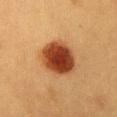The tile uses cross-polarized illumination.
A 15 mm close-up extracted from a 3D total-body photography capture.
The subject is a female in their 40s.
Measured at roughly 5 mm in maximum diameter.
The lesion is located on the front of the torso.
The lesion-visualizer software estimated an area of roughly 16 mm² and two-axis asymmetry of about 0.1. The software also gave a lesion–skin lightness drop of about 18. And it measured an automated nevus-likeness rating near 100 out of 100.